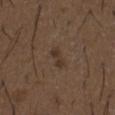subject: male, aged approximately 50
imaging modality: total-body-photography crop, ~15 mm field of view
site: the front of the torso